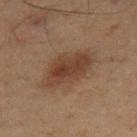notes — catalogued during a skin exam; not biopsied | tile lighting — cross-polarized illumination | image-analysis metrics — a lesion color around L≈29 a*≈15 b*≈22 in CIELAB, a lesion–skin lightness drop of about 7, and a lesion-to-skin contrast of about 8 (normalized; higher = more distinct); a border-irregularity index near 2/10, internal color variation of about 3.5 on a 0–10 scale, and a peripheral color-asymmetry measure near 1; a detector confidence of about 100 out of 100 that the crop contains a lesion | image source — ~15 mm crop, total-body skin-cancer survey | patient — male, aged around 50 | location — the chest.